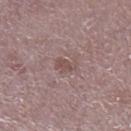biopsy status: total-body-photography surveillance lesion; no biopsy | subject: male, roughly 50 years of age | diameter: about 2.5 mm | location: the leg | image: ~15 mm tile from a whole-body skin photo.